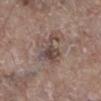| field | value |
|---|---|
| workup | total-body-photography surveillance lesion; no biopsy |
| image | 15 mm crop, total-body photography |
| patient | male, aged 63–67 |
| body site | the right lower leg |